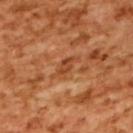workup = imaged on a skin check; not biopsied
image = 15 mm crop, total-body photography
site = the upper back
subject = female, aged 53–57
illumination = cross-polarized